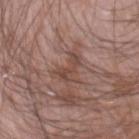This lesion was catalogued during total-body skin photography and was not selected for biopsy. Measured at roughly 4 mm in maximum diameter. The patient is a male approximately 60 years of age. Captured under white-light illumination. Located on the right forearm. A 15 mm close-up tile from a total-body photography series done for melanoma screening.